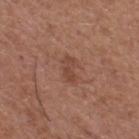The lesion was tiled from a total-body skin photograph and was not biopsied. The patient is a male aged around 75. Cropped from a total-body skin-imaging series; the visible field is about 15 mm. Imaged with white-light lighting. The lesion is on the right thigh. Measured at roughly 3 mm in maximum diameter. The total-body-photography lesion software estimated a lesion-to-skin contrast of about 6 (normalized; higher = more distinct). It also reported border irregularity of about 4 on a 0–10 scale, a within-lesion color-variation index near 2/10, and peripheral color asymmetry of about 0.5. The analysis additionally found an automated nevus-likeness rating near 0 out of 100 and a detector confidence of about 100 out of 100 that the crop contains a lesion.